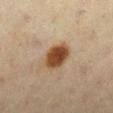Q: Was a biopsy performed?
A: no biopsy performed (imaged during a skin exam)
Q: How large is the lesion?
A: ≈3.5 mm
Q: Patient demographics?
A: female, in their mid- to late 60s
Q: Automated lesion metrics?
A: a footprint of about 8.5 mm² and an eccentricity of roughly 0.6; roughly 15 lightness units darker than nearby skin; a nevus-likeness score of about 100/100 and a detector confidence of about 100 out of 100 that the crop contains a lesion
Q: What kind of image is this?
A: 15 mm crop, total-body photography
Q: Lesion location?
A: the right lower leg
Q: Illumination type?
A: cross-polarized illumination